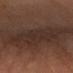Q: Was a biopsy performed?
A: imaged on a skin check; not biopsied
Q: Lesion location?
A: the left forearm
Q: How large is the lesion?
A: ~8 mm (longest diameter)
Q: Who is the patient?
A: male, in their 60s
Q: What lighting was used for the tile?
A: cross-polarized illumination
Q: What kind of image is this?
A: ~15 mm tile from a whole-body skin photo